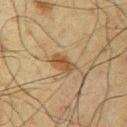follow-up = total-body-photography surveillance lesion; no biopsy | size = about 3 mm | subject = male, aged 58–62 | TBP lesion metrics = a lesion color around L≈39 a*≈14 b*≈29 in CIELAB and a normalized lesion–skin contrast near 7.5; a border-irregularity index near 2.5/10 and peripheral color asymmetry of about 1.5 | image = ~15 mm crop, total-body skin-cancer survey | lighting = cross-polarized illumination | location = the arm.